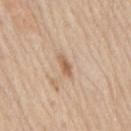Notes:
* biopsy status: total-body-photography surveillance lesion; no biopsy
* acquisition: ~15 mm tile from a whole-body skin photo
* lesion diameter: ≈3 mm
* anatomic site: the back
* patient: male, aged around 60
* tile lighting: white-light